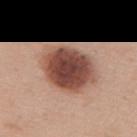Part of a total-body skin-imaging series; this lesion was reviewed on a skin check and was not flagged for biopsy. This is a white-light tile. The recorded lesion diameter is about 6.5 mm. This image is a 15 mm lesion crop taken from a total-body photograph. The lesion is on the upper back. A female subject in their 50s.